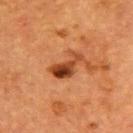– notes · no biopsy performed (imaged during a skin exam)
– tile lighting · cross-polarized
– subject · female, roughly 55 years of age
– anatomic site · the upper back
– acquisition · ~15 mm crop, total-body skin-cancer survey